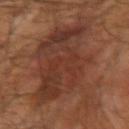workup: total-body-photography surveillance lesion; no biopsy | image source: ~15 mm tile from a whole-body skin photo | lighting: cross-polarized illumination | location: the right upper arm | size: ≈13 mm | patient: male, approximately 65 years of age.